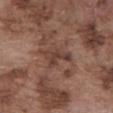{"biopsy_status": "not biopsied; imaged during a skin examination", "lighting": "white-light", "image": {"source": "total-body photography crop", "field_of_view_mm": 15}, "automated_metrics": {"border_irregularity_0_10": 5.0, "color_variation_0_10": 2.5, "peripheral_color_asymmetry": 1.0, "nevus_likeness_0_100": 0, "lesion_detection_confidence_0_100": 95}, "patient": {"sex": "male", "age_approx": 75}, "site": "abdomen", "lesion_size": {"long_diameter_mm_approx": 4.0}}